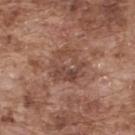Impression: The lesion was tiled from a total-body skin photograph and was not biopsied.